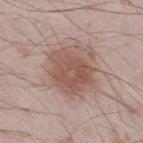{
  "biopsy_status": "not biopsied; imaged during a skin examination"
}